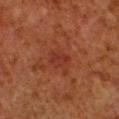A male patient roughly 80 years of age.
The lesion is located on the left lower leg.
Measured at roughly 2.5 mm in maximum diameter.
The lesion-visualizer software estimated a lesion area of about 4 mm², a shape eccentricity near 0.75, and two-axis asymmetry of about 0.25. The software also gave an average lesion color of about L≈26 a*≈24 b*≈25 (CIELAB), about 5 CIELAB-L* units darker than the surrounding skin, and a lesion-to-skin contrast of about 5.5 (normalized; higher = more distinct). The software also gave a border-irregularity index near 2/10, a within-lesion color-variation index near 1.5/10, and peripheral color asymmetry of about 0.5.
This is a cross-polarized tile.
A 15 mm close-up tile from a total-body photography series done for melanoma screening.